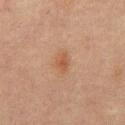| feature | finding |
|---|---|
| biopsy status | no biopsy performed (imaged during a skin exam) |
| image | total-body-photography crop, ~15 mm field of view |
| size | about 3 mm |
| patient | male, aged 63 to 67 |
| anatomic site | the mid back |
| lighting | cross-polarized |
| TBP lesion metrics | an average lesion color of about L≈43 a*≈18 b*≈28 (CIELAB) and about 6 CIELAB-L* units darker than the surrounding skin; a nevus-likeness score of about 75/100 |